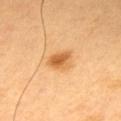Imaged during a routine full-body skin examination; the lesion was not biopsied and no histopathology is available. Captured under cross-polarized illumination. A lesion tile, about 15 mm wide, cut from a 3D total-body photograph. The patient is a male roughly 50 years of age. Located on the upper back. Automated image analysis of the tile measured an outline eccentricity of about 0.8 (0 = round, 1 = elongated). The software also gave a border-irregularity rating of about 2/10 and a within-lesion color-variation index near 5/10. The analysis additionally found a classifier nevus-likeness of about 100/100 and lesion-presence confidence of about 100/100.